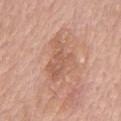Clinical impression: Recorded during total-body skin imaging; not selected for excision or biopsy. Acquisition and patient details: Measured at roughly 6.5 mm in maximum diameter. The lesion is located on the chest. Imaged with white-light lighting. A male subject, about 80 years old. A 15 mm close-up extracted from a 3D total-body photography capture.